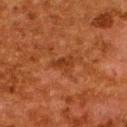The lesion was tiled from a total-body skin photograph and was not biopsied. Cropped from a whole-body photographic skin survey; the tile spans about 15 mm. From the back. This is a cross-polarized tile. The lesion-visualizer software estimated a footprint of about 3.5 mm², an eccentricity of roughly 0.8, and two-axis asymmetry of about 0.4. The software also gave lesion-presence confidence of about 100/100. The patient is a female aged 48–52. The recorded lesion diameter is about 2.5 mm.